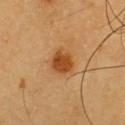Q: Was this lesion biopsied?
A: imaged on a skin check; not biopsied
Q: What did automated image analysis measure?
A: an area of roughly 6.5 mm² and an eccentricity of roughly 0.25; a border-irregularity rating of about 1.5/10 and radial color variation of about 1; a nevus-likeness score of about 100/100 and a detector confidence of about 100 out of 100 that the crop contains a lesion
Q: Illumination type?
A: cross-polarized illumination
Q: What is the imaging modality?
A: ~15 mm crop, total-body skin-cancer survey
Q: What is the anatomic site?
A: the chest
Q: Patient demographics?
A: male, aged approximately 60